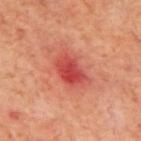Captured under cross-polarized illumination. A region of skin cropped from a whole-body photographic capture, roughly 15 mm wide. Located on the mid back. A male subject, approximately 70 years of age. The lesion-visualizer software estimated two-axis asymmetry of about 0.2. The software also gave a border-irregularity index near 2.5/10, a color-variation rating of about 5/10, and radial color variation of about 1.5. And it measured a nevus-likeness score of about 0/100 and a detector confidence of about 100 out of 100 that the crop contains a lesion. Longest diameter approximately 4.5 mm.